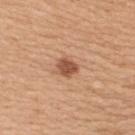The lesion was photographed on a routine skin check and not biopsied; there is no pathology result.
The lesion-visualizer software estimated an outline eccentricity of about 0.6 (0 = round, 1 = elongated) and two-axis asymmetry of about 0.2. The software also gave a border-irregularity rating of about 2/10.
A close-up tile cropped from a whole-body skin photograph, about 15 mm across.
Located on the upper back.
The tile uses white-light illumination.
About 2.5 mm across.
The subject is a female about 30 years old.